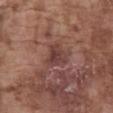TBP lesion metrics: a footprint of about 5.5 mm² and a shape eccentricity near 0.35; a lesion color around L≈40 a*≈21 b*≈22 in CIELAB, roughly 8 lightness units darker than nearby skin, and a normalized border contrast of about 7 | imaging modality: total-body-photography crop, ~15 mm field of view | anatomic site: the abdomen | lighting: white-light | subject: male, roughly 75 years of age | size: about 3 mm.